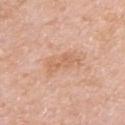Assessment: The lesion was photographed on a routine skin check and not biopsied; there is no pathology result. Background: Automated image analysis of the tile measured a lesion color around L≈64 a*≈21 b*≈34 in CIELAB, about 7 CIELAB-L* units darker than the surrounding skin, and a normalized lesion–skin contrast near 5.5. And it measured a lesion-detection confidence of about 100/100. A 15 mm crop from a total-body photograph taken for skin-cancer surveillance. On the right upper arm. A female patient, in their mid- to late 50s. The lesion's longest dimension is about 4.5 mm. Captured under white-light illumination.